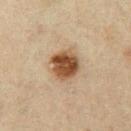Q: Was a biopsy performed?
A: no biopsy performed (imaged during a skin exam)
Q: What did automated image analysis measure?
A: an area of roughly 11 mm², a shape eccentricity near 0.6, and a shape-asymmetry score of about 0.15 (0 = symmetric); an average lesion color of about L≈43 a*≈16 b*≈30 (CIELAB), roughly 15 lightness units darker than nearby skin, and a normalized border contrast of about 11.5; a border-irregularity index near 2/10, a within-lesion color-variation index near 5.5/10, and radial color variation of about 1.5; a classifier nevus-likeness of about 100/100 and lesion-presence confidence of about 100/100
Q: How was this image acquired?
A: total-body-photography crop, ~15 mm field of view
Q: Where on the body is the lesion?
A: the left upper arm
Q: How large is the lesion?
A: about 4 mm
Q: Patient demographics?
A: male, in their mid- to late 40s
Q: How was the tile lit?
A: cross-polarized